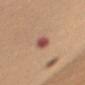This lesion was catalogued during total-body skin photography and was not selected for biopsy. A region of skin cropped from a whole-body photographic capture, roughly 15 mm wide. On the left upper arm. The patient is a female in their 60s. Approximately 2.5 mm at its widest.